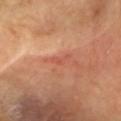• notes — no biopsy performed (imaged during a skin exam)
• size — ~2.5 mm (longest diameter)
• image source — 15 mm crop, total-body photography
• body site — the head or neck
• subject — female, aged around 70